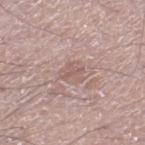follow-up=catalogued during a skin exam; not biopsied
imaging modality=~15 mm tile from a whole-body skin photo
subject=male, roughly 60 years of age
body site=the left thigh